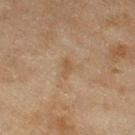| field | value |
|---|---|
| biopsy status | catalogued during a skin exam; not biopsied |
| subject | female, aged 58–62 |
| acquisition | ~15 mm tile from a whole-body skin photo |
| illumination | cross-polarized |
| TBP lesion metrics | a mean CIELAB color near L≈49 a*≈15 b*≈31 and roughly 6 lightness units darker than nearby skin; border irregularity of about 4 on a 0–10 scale, a within-lesion color-variation index near 0.5/10, and a peripheral color-asymmetry measure near 0 |
| lesion diameter | ≈2.5 mm |
| anatomic site | the right thigh |